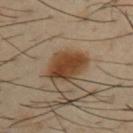{"biopsy_status": "not biopsied; imaged during a skin examination", "automated_metrics": {"area_mm2_approx": 12.0, "eccentricity": 0.75, "shape_asymmetry": 0.2, "cielab_L": 40, "cielab_a": 18, "cielab_b": 31, "vs_skin_darker_L": 12.0, "vs_skin_contrast_norm": 11.0, "border_irregularity_0_10": 2.0, "nevus_likeness_0_100": 100, "lesion_detection_confidence_0_100": 100}, "lesion_size": {"long_diameter_mm_approx": 4.5}, "lighting": "cross-polarized", "image": {"source": "total-body photography crop", "field_of_view_mm": 15}, "site": "left upper arm", "patient": {"sex": "male", "age_approx": 40}}